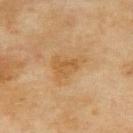Imaged during a routine full-body skin examination; the lesion was not biopsied and no histopathology is available. The subject is a female aged 58–62. Cropped from a whole-body photographic skin survey; the tile spans about 15 mm. From the back. Imaged with cross-polarized lighting. The recorded lesion diameter is about 4 mm.